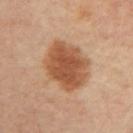Impression:
The lesion was tiled from a total-body skin photograph and was not biopsied.
Image and clinical context:
A close-up tile cropped from a whole-body skin photograph, about 15 mm across. Located on the left upper arm. This is a cross-polarized tile. Longest diameter approximately 6.5 mm. The subject is a male aged 63 to 67. The total-body-photography lesion software estimated roughly 13 lightness units darker than nearby skin and a normalized lesion–skin contrast near 9. The software also gave internal color variation of about 4.5 on a 0–10 scale and radial color variation of about 1.5. The analysis additionally found a classifier nevus-likeness of about 95/100 and a lesion-detection confidence of about 100/100.